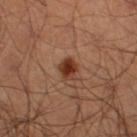<tbp_lesion>
<biopsy_status>not biopsied; imaged during a skin examination</biopsy_status>
<image>
  <source>total-body photography crop</source>
  <field_of_view_mm>15</field_of_view_mm>
</image>
<lighting>cross-polarized</lighting>
<site>left lower leg</site>
<patient>
  <sex>male</sex>
  <age_approx>55</age_approx>
</patient>
<lesion_size>
  <long_diameter_mm_approx>2.5</long_diameter_mm_approx>
</lesion_size>
</tbp_lesion>